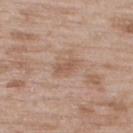workup: total-body-photography surveillance lesion; no biopsy | size: ~2.5 mm (longest diameter) | image source: 15 mm crop, total-body photography | body site: the upper back | illumination: white-light illumination | subject: male, about 50 years old.